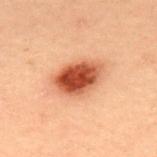<case>
<biopsy_status>not biopsied; imaged during a skin examination</biopsy_status>
<site>back</site>
<patient>
  <sex>male</sex>
  <age_approx>55</age_approx>
</patient>
<image>
  <source>total-body photography crop</source>
  <field_of_view_mm>15</field_of_view_mm>
</image>
</case>